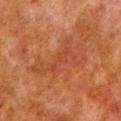Q: Was this lesion biopsied?
A: catalogued during a skin exam; not biopsied
Q: What is the imaging modality?
A: ~15 mm crop, total-body skin-cancer survey
Q: Automated lesion metrics?
A: an area of roughly 14 mm² and two-axis asymmetry of about 0.55; a border-irregularity index near 9/10, internal color variation of about 3 on a 0–10 scale, and peripheral color asymmetry of about 1
Q: What lighting was used for the tile?
A: cross-polarized illumination
Q: Patient demographics?
A: male, aged approximately 80
Q: Lesion location?
A: the left lower leg
Q: How large is the lesion?
A: ~8 mm (longest diameter)